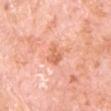Automated tile analysis of the lesion measured a normalized lesion–skin contrast near 6.5. It also reported a color-variation rating of about 2/10 and radial color variation of about 1. Longest diameter approximately 2.5 mm. Located on the front of the torso. A male subject, in their 80s. A 15 mm close-up tile from a total-body photography series done for melanoma screening.